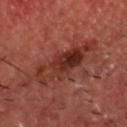Recorded during total-body skin imaging; not selected for excision or biopsy.
About 6.5 mm across.
Located on the head or neck.
The subject is a male roughly 60 years of age.
Automated image analysis of the tile measured a lesion area of about 19 mm², a shape eccentricity near 0.8, and a shape-asymmetry score of about 0.3 (0 = symmetric). And it measured about 7 CIELAB-L* units darker than the surrounding skin.
This is a cross-polarized tile.
A roughly 15 mm field-of-view crop from a total-body skin photograph.